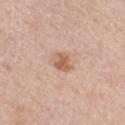Imaged during a routine full-body skin examination; the lesion was not biopsied and no histopathology is available.
A 15 mm close-up extracted from a 3D total-body photography capture.
The patient is a male aged 58 to 62.
The lesion's longest dimension is about 3 mm.
From the left upper arm.
Automated tile analysis of the lesion measured an average lesion color of about L≈61 a*≈20 b*≈32 (CIELAB), about 10 CIELAB-L* units darker than the surrounding skin, and a normalized lesion–skin contrast near 7. The analysis additionally found a border-irregularity index near 2/10, a within-lesion color-variation index near 3.5/10, and radial color variation of about 1. It also reported lesion-presence confidence of about 100/100.